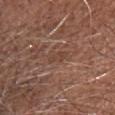Assessment:
The lesion was photographed on a routine skin check and not biopsied; there is no pathology result.
Acquisition and patient details:
From the head or neck. Automated image analysis of the tile measured a lesion color around L≈42 a*≈20 b*≈27 in CIELAB and about 6 CIELAB-L* units darker than the surrounding skin. The software also gave a border-irregularity index near 3/10, a color-variation rating of about 0.5/10, and peripheral color asymmetry of about 0.5. And it measured a detector confidence of about 55 out of 100 that the crop contains a lesion. The subject is a male approximately 70 years of age. This is a white-light tile. A roughly 15 mm field-of-view crop from a total-body skin photograph. The recorded lesion diameter is about 2.5 mm.